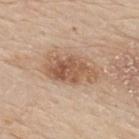Impression: Part of a total-body skin-imaging series; this lesion was reviewed on a skin check and was not flagged for biopsy. Clinical summary: On the upper back. Measured at roughly 6.5 mm in maximum diameter. This image is a 15 mm lesion crop taken from a total-body photograph. Automated tile analysis of the lesion measured lesion-presence confidence of about 100/100. The patient is a male aged around 80.